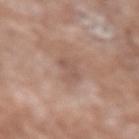Findings:
• notes — imaged on a skin check; not biopsied
• body site — the left upper arm
• patient — male, roughly 60 years of age
• image source — 15 mm crop, total-body photography